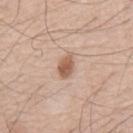Impression: Recorded during total-body skin imaging; not selected for excision or biopsy. Clinical summary: Captured under white-light illumination. The lesion is located on the mid back. This image is a 15 mm lesion crop taken from a total-body photograph. The patient is a male about 75 years old.